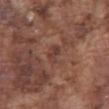| key | value |
|---|---|
| biopsy status | catalogued during a skin exam; not biopsied |
| location | the abdomen |
| diameter | ≈2.5 mm |
| image source | ~15 mm tile from a whole-body skin photo |
| image-analysis metrics | a lesion color around L≈39 a*≈20 b*≈24 in CIELAB, a lesion–skin lightness drop of about 7, and a lesion-to-skin contrast of about 6 (normalized; higher = more distinct); an automated nevus-likeness rating near 0 out of 100 |
| patient | male, aged 73 to 77 |
| illumination | white-light |